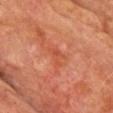* follow-up — total-body-photography surveillance lesion; no biopsy
* lighting — cross-polarized
* size — about 3 mm
* image-analysis metrics — a within-lesion color-variation index near 0/10 and radial color variation of about 0; a classifier nevus-likeness of about 0/100 and lesion-presence confidence of about 100/100
* location — the chest
* subject — male, aged approximately 75
* image source — ~15 mm tile from a whole-body skin photo